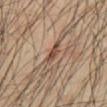Q: Is there a histopathology result?
A: no biopsy performed (imaged during a skin exam)
Q: Patient demographics?
A: roughly 65 years of age
Q: What is the imaging modality?
A: ~15 mm tile from a whole-body skin photo
Q: Where on the body is the lesion?
A: the abdomen